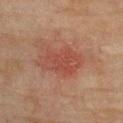Assessment: Imaged during a routine full-body skin examination; the lesion was not biopsied and no histopathology is available. Acquisition and patient details: The subject is a male about 75 years old. Captured under cross-polarized illumination. Automated tile analysis of the lesion measured a classifier nevus-likeness of about 45/100 and a lesion-detection confidence of about 100/100. Located on the upper back. A 15 mm close-up extracted from a 3D total-body photography capture.